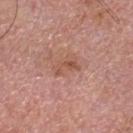Q: Was this lesion biopsied?
A: catalogued during a skin exam; not biopsied